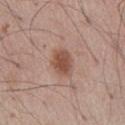The lesion was tiled from a total-body skin photograph and was not biopsied. Measured at roughly 3.5 mm in maximum diameter. The lesion is on the abdomen. A male patient in their mid- to late 50s. A 15 mm crop from a total-body photograph taken for skin-cancer surveillance. An algorithmic analysis of the crop reported a mean CIELAB color near L≈50 a*≈21 b*≈27 and a normalized border contrast of about 8.5.